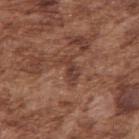Captured during whole-body skin photography for melanoma surveillance; the lesion was not biopsied.
On the right upper arm.
The patient is a male aged 73–77.
About 3.5 mm across.
A close-up tile cropped from a whole-body skin photograph, about 15 mm across.
The lesion-visualizer software estimated an outline eccentricity of about 0.85 (0 = round, 1 = elongated) and a symmetry-axis asymmetry near 0.5. The analysis additionally found a border-irregularity rating of about 6/10, a within-lesion color-variation index near 2.5/10, and peripheral color asymmetry of about 1.